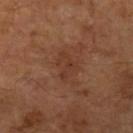Notes:
* follow-up · catalogued during a skin exam; not biopsied
* size · ≈3.5 mm
* acquisition · ~15 mm tile from a whole-body skin photo
* anatomic site · the left upper arm
* patient · male, aged 63–67